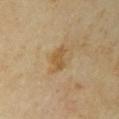The lesion was tiled from a total-body skin photograph and was not biopsied. A female subject approximately 35 years of age. The lesion is located on the chest. The tile uses cross-polarized illumination. A roughly 15 mm field-of-view crop from a total-body skin photograph. Longest diameter approximately 3.5 mm.